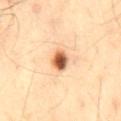Assessment: Recorded during total-body skin imaging; not selected for excision or biopsy. Acquisition and patient details: A male subject roughly 40 years of age. On the mid back. The recorded lesion diameter is about 3 mm. A 15 mm close-up extracted from a 3D total-body photography capture. Imaged with cross-polarized lighting.